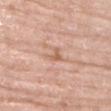The lesion was tiled from a total-body skin photograph and was not biopsied.
The subject is a female about 70 years old.
The lesion is on the right upper arm.
About 3 mm across.
The tile uses white-light illumination.
This image is a 15 mm lesion crop taken from a total-body photograph.